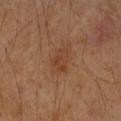notes: no biopsy performed (imaged during a skin exam) | automated lesion analysis: a border-irregularity index near 4.5/10 and a color-variation rating of about 3/10 | patient: female, about 40 years old | body site: the right forearm | tile lighting: cross-polarized | lesion diameter: ~3 mm (longest diameter) | imaging modality: total-body-photography crop, ~15 mm field of view.